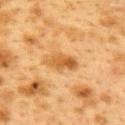biopsy status: catalogued during a skin exam; not biopsied | TBP lesion metrics: roughly 10 lightness units darker than nearby skin; internal color variation of about 5 on a 0–10 scale; an automated nevus-likeness rating near 0 out of 100 and a lesion-detection confidence of about 100/100 | location: the upper back | image source: 15 mm crop, total-body photography | patient: female, aged 38–42 | diameter: about 4 mm.